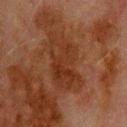notes: total-body-photography surveillance lesion; no biopsy